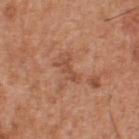Captured during whole-body skin photography for melanoma surveillance; the lesion was not biopsied.
A male patient roughly 55 years of age.
Measured at roughly 3 mm in maximum diameter.
A close-up tile cropped from a whole-body skin photograph, about 15 mm across.
This is a white-light tile.
The lesion is on the upper back.